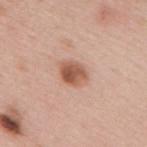Assessment: Part of a total-body skin-imaging series; this lesion was reviewed on a skin check and was not flagged for biopsy. Image and clinical context: An algorithmic analysis of the crop reported a footprint of about 7 mm², an eccentricity of roughly 0.65, and a symmetry-axis asymmetry near 0.2. The software also gave an average lesion color of about L≈56 a*≈23 b*≈31 (CIELAB) and a normalized border contrast of about 9. The analysis additionally found border irregularity of about 2 on a 0–10 scale, internal color variation of about 5.5 on a 0–10 scale, and peripheral color asymmetry of about 2. A roughly 15 mm field-of-view crop from a total-body skin photograph. The patient is a female aged around 40. The lesion's longest dimension is about 3.5 mm. Located on the upper back.